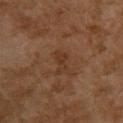<record>
<biopsy_status>not biopsied; imaged during a skin examination</biopsy_status>
<automated_metrics>
  <cielab_L>30</cielab_L>
  <cielab_a>17</cielab_a>
  <cielab_b>27</cielab_b>
  <vs_skin_darker_L>5.0</vs_skin_darker_L>
  <vs_skin_contrast_norm>6.0</vs_skin_contrast_norm>
</automated_metrics>
<lesion_size>
  <long_diameter_mm_approx>3.0</long_diameter_mm_approx>
</lesion_size>
<lighting>cross-polarized</lighting>
<image>
  <source>total-body photography crop</source>
  <field_of_view_mm>15</field_of_view_mm>
</image>
<patient>
  <sex>female</sex>
  <age_approx>60</age_approx>
</patient>
<site>back</site>
</record>